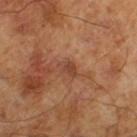biopsy_status: not biopsied; imaged during a skin examination
lesion_size:
  long_diameter_mm_approx: 2.5
automated_metrics:
  area_mm2_approx: 3.0
  shape_asymmetry: 0.2
  border_irregularity_0_10: 2.0
  color_variation_0_10: 1.5
  peripheral_color_asymmetry: 0.5
lighting: cross-polarized
patient:
  sex: male
  age_approx: 65
image:
  source: total-body photography crop
  field_of_view_mm: 15